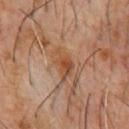{
  "image": {
    "source": "total-body photography crop",
    "field_of_view_mm": 15
  },
  "patient": {
    "sex": "male",
    "age_approx": 60
  },
  "site": "chest",
  "lesion_size": {
    "long_diameter_mm_approx": 4.0
  },
  "lighting": "cross-polarized"
}